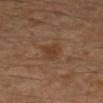{
  "image": {
    "source": "total-body photography crop",
    "field_of_view_mm": 15
  },
  "patient": {
    "sex": "male",
    "age_approx": 30
  },
  "site": "left leg"
}